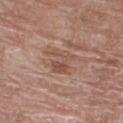{
  "biopsy_status": "not biopsied; imaged during a skin examination",
  "automated_metrics": {
    "area_mm2_approx": 6.0,
    "eccentricity": 0.65,
    "shape_asymmetry": 0.4,
    "color_variation_0_10": 4.0,
    "peripheral_color_asymmetry": 1.5,
    "nevus_likeness_0_100": 0,
    "lesion_detection_confidence_0_100": 100
  },
  "image": {
    "source": "total-body photography crop",
    "field_of_view_mm": 15
  },
  "lesion_size": {
    "long_diameter_mm_approx": 3.5
  },
  "site": "right thigh",
  "patient": {
    "sex": "female",
    "age_approx": 70
  }
}